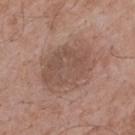Impression: Imaged during a routine full-body skin examination; the lesion was not biopsied and no histopathology is available. Context: Captured under white-light illumination. From the upper back. A male patient, approximately 70 years of age. A 15 mm crop from a total-body photograph taken for skin-cancer surveillance. The recorded lesion diameter is about 7 mm.